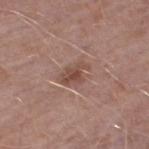Case summary:
* biopsy status: catalogued during a skin exam; not biopsied
* imaging modality: total-body-photography crop, ~15 mm field of view
* body site: the leg
* diameter: about 3.5 mm
* subject: male, aged 48–52
* lighting: white-light